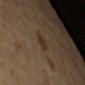Assessment: Part of a total-body skin-imaging series; this lesion was reviewed on a skin check and was not flagged for biopsy. Clinical summary: A roughly 15 mm field-of-view crop from a total-body skin photograph. The patient is a male aged 63 to 67.